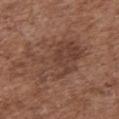The lesion was tiled from a total-body skin photograph and was not biopsied.
A female patient about 75 years old.
The recorded lesion diameter is about 7 mm.
The lesion is on the front of the torso.
A roughly 15 mm field-of-view crop from a total-body skin photograph.
Captured under white-light illumination.
The lesion-visualizer software estimated an area of roughly 24 mm², a shape eccentricity near 0.75, and a symmetry-axis asymmetry near 0.45. The analysis additionally found a lesion color around L≈41 a*≈19 b*≈26 in CIELAB, a lesion–skin lightness drop of about 7, and a lesion-to-skin contrast of about 6 (normalized; higher = more distinct).